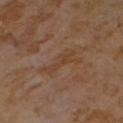The tile uses cross-polarized illumination. The lesion is on the front of the torso. A lesion tile, about 15 mm wide, cut from a 3D total-body photograph. The patient is a female aged around 55. About 5 mm across. An algorithmic analysis of the crop reported an area of roughly 7 mm², an outline eccentricity of about 0.95 (0 = round, 1 = elongated), and a shape-asymmetry score of about 0.45 (0 = symmetric). The software also gave an average lesion color of about L≈42 a*≈18 b*≈29 (CIELAB), about 5 CIELAB-L* units darker than the surrounding skin, and a normalized border contrast of about 5. And it measured a within-lesion color-variation index near 2/10 and radial color variation of about 1.